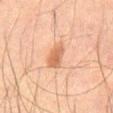Findings:
- biopsy status · catalogued during a skin exam; not biopsied
- tile lighting · cross-polarized
- anatomic site · the abdomen
- acquisition · total-body-photography crop, ~15 mm field of view
- subject · male, about 45 years old
- TBP lesion metrics · an average lesion color of about L≈53 a*≈21 b*≈31 (CIELAB), about 10 CIELAB-L* units darker than the surrounding skin, and a lesion-to-skin contrast of about 7 (normalized; higher = more distinct); a border-irregularity index near 2.5/10, internal color variation of about 2.5 on a 0–10 scale, and radial color variation of about 0.5